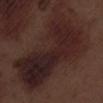Clinical impression:
Recorded during total-body skin imaging; not selected for excision or biopsy.
Context:
The total-body-photography lesion software estimated a lesion area of about 60 mm², an eccentricity of roughly 0.85, and two-axis asymmetry of about 0.4. And it measured internal color variation of about 7 on a 0–10 scale and peripheral color asymmetry of about 2.5. And it measured an automated nevus-likeness rating near 0 out of 100 and a detector confidence of about 75 out of 100 that the crop contains a lesion. The lesion is located on the left lower leg. Approximately 14 mm at its widest. A 15 mm close-up tile from a total-body photography series done for melanoma screening. A male subject about 70 years old.